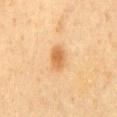This lesion was catalogued during total-body skin photography and was not selected for biopsy.
A male subject, approximately 60 years of age.
Automated image analysis of the tile measured a shape eccentricity near 0.75 and a symmetry-axis asymmetry near 0.2. The analysis additionally found a mean CIELAB color near L≈54 a*≈20 b*≈37 and a normalized lesion–skin contrast near 7.5.
From the mid back.
This image is a 15 mm lesion crop taken from a total-body photograph.